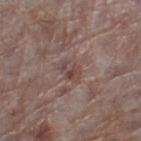No biopsy was performed on this lesion — it was imaged during a full skin examination and was not determined to be concerning. A 15 mm crop from a total-body photograph taken for skin-cancer surveillance. Imaged with white-light lighting. The lesion is on the left thigh. An algorithmic analysis of the crop reported an outline eccentricity of about 0.7 (0 = round, 1 = elongated) and two-axis asymmetry of about 0.2. And it measured a lesion color around L≈45 a*≈17 b*≈20 in CIELAB, a lesion–skin lightness drop of about 7, and a lesion-to-skin contrast of about 5.5 (normalized; higher = more distinct). The patient is a female aged approximately 85 or older.